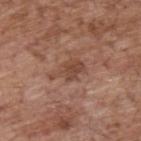Q: What did automated image analysis measure?
A: an area of roughly 7 mm², an outline eccentricity of about 0.85 (0 = round, 1 = elongated), and a shape-asymmetry score of about 0.4 (0 = symmetric); an average lesion color of about L≈46 a*≈21 b*≈28 (CIELAB), a lesion–skin lightness drop of about 8, and a normalized border contrast of about 6.5; a classifier nevus-likeness of about 0/100 and a detector confidence of about 100 out of 100 that the crop contains a lesion
Q: Lesion size?
A: about 4.5 mm
Q: What is the anatomic site?
A: the upper back
Q: How was the tile lit?
A: white-light illumination
Q: Patient demographics?
A: male, aged approximately 70
Q: How was this image acquired?
A: 15 mm crop, total-body photography